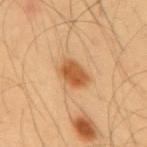follow-up=no biopsy performed (imaged during a skin exam)
image-analysis metrics=an average lesion color of about L≈55 a*≈23 b*≈41 (CIELAB) and a normalized border contrast of about 9; border irregularity of about 2 on a 0–10 scale, internal color variation of about 3 on a 0–10 scale, and a peripheral color-asymmetry measure near 1; an automated nevus-likeness rating near 100 out of 100
lesion diameter=about 3.5 mm
subject=male, aged around 55
site=the back
acquisition=15 mm crop, total-body photography